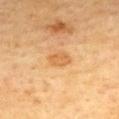notes=catalogued during a skin exam; not biopsied | imaging modality=total-body-photography crop, ~15 mm field of view | location=the mid back | illumination=cross-polarized | patient=female, approximately 55 years of age | automated metrics=a footprint of about 4 mm² and an eccentricity of roughly 0.85; a classifier nevus-likeness of about 15/100 and lesion-presence confidence of about 100/100.